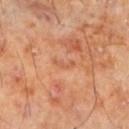A male patient roughly 70 years of age.
The lesion is on the left lower leg.
The total-body-photography lesion software estimated a lesion color around L≈56 a*≈26 b*≈36 in CIELAB and a normalized border contrast of about 4.5.
Imaged with cross-polarized lighting.
A lesion tile, about 15 mm wide, cut from a 3D total-body photograph.